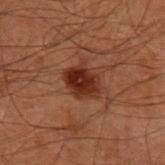Impression:
Part of a total-body skin-imaging series; this lesion was reviewed on a skin check and was not flagged for biopsy.
Background:
A region of skin cropped from a whole-body photographic capture, roughly 15 mm wide. The lesion is located on the arm. The lesion's longest dimension is about 3.5 mm. A male patient in their 60s. Imaged with cross-polarized lighting.